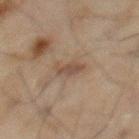image:
  source: total-body photography crop
  field_of_view_mm: 15
site: chest
patient:
  sex: male
  age_approx: 70